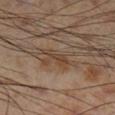follow-up: catalogued during a skin exam; not biopsied
imaging modality: 15 mm crop, total-body photography
lesion diameter: ~3.5 mm (longest diameter)
subject: male, aged 53–57
illumination: cross-polarized
body site: the right lower leg